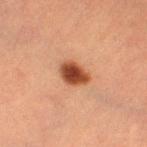Clinical impression:
This lesion was catalogued during total-body skin photography and was not selected for biopsy.
Image and clinical context:
The lesion is on the left thigh. A lesion tile, about 15 mm wide, cut from a 3D total-body photograph. The patient is a female in their mid- to late 50s. The recorded lesion diameter is about 3.5 mm. The lesion-visualizer software estimated an outline eccentricity of about 0.7 (0 = round, 1 = elongated) and two-axis asymmetry of about 0.2. The analysis additionally found a lesion color around L≈38 a*≈23 b*≈30 in CIELAB, about 15 CIELAB-L* units darker than the surrounding skin, and a normalized border contrast of about 12. The software also gave a border-irregularity index near 2/10, a color-variation rating of about 4.5/10, and a peripheral color-asymmetry measure near 1.